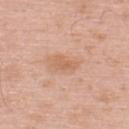Case summary:
• lesion size — ~3 mm (longest diameter)
• patient — male, roughly 60 years of age
• illumination — white-light illumination
• automated lesion analysis — a lesion color around L≈63 a*≈22 b*≈33 in CIELAB, about 7 CIELAB-L* units darker than the surrounding skin, and a lesion-to-skin contrast of about 5.5 (normalized; higher = more distinct); a lesion-detection confidence of about 100/100
• anatomic site — the back
• image — total-body-photography crop, ~15 mm field of view